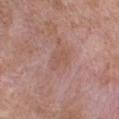Notes:
• notes — imaged on a skin check; not biopsied
• size — about 3 mm
• body site — the front of the torso
• patient — male, in their 50s
• tile lighting — white-light illumination
• image — ~15 mm crop, total-body skin-cancer survey